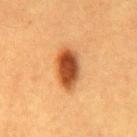{"image": {"source": "total-body photography crop", "field_of_view_mm": 15}, "patient": {"sex": "male", "age_approx": 60}, "site": "front of the torso", "lesion_size": {"long_diameter_mm_approx": 5.0}, "lighting": "cross-polarized"}